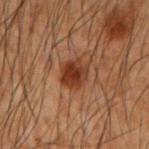| key | value |
|---|---|
| patient | male, aged around 50 |
| lighting | cross-polarized illumination |
| size | ≈3.5 mm |
| acquisition | 15 mm crop, total-body photography |
| anatomic site | the arm |
| TBP lesion metrics | a shape eccentricity near 0.6; an average lesion color of about L≈28 a*≈20 b*≈26 (CIELAB), about 10 CIELAB-L* units darker than the surrounding skin, and a normalized lesion–skin contrast near 10 |